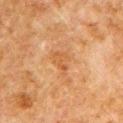Part of a total-body skin-imaging series; this lesion was reviewed on a skin check and was not flagged for biopsy. The subject is a male in their 80s. The lesion is located on the left upper arm. Automated image analysis of the tile measured an area of roughly 4.5 mm². The software also gave a mean CIELAB color near L≈45 a*≈21 b*≈35, a lesion–skin lightness drop of about 6, and a lesion-to-skin contrast of about 5 (normalized; higher = more distinct). The software also gave a border-irregularity rating of about 4.5/10 and peripheral color asymmetry of about 1. A 15 mm close-up extracted from a 3D total-body photography capture. Measured at roughly 3 mm in maximum diameter.